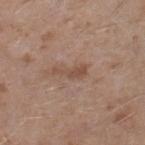lesion diameter — about 3.5 mm
automated metrics — a footprint of about 3.5 mm², an outline eccentricity of about 0.95 (0 = round, 1 = elongated), and a shape-asymmetry score of about 0.55 (0 = symmetric); an automated nevus-likeness rating near 0 out of 100
body site — the right lower leg
patient — male, aged approximately 45
image — ~15 mm tile from a whole-body skin photo
tile lighting — white-light